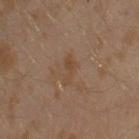notes: imaged on a skin check; not biopsied
body site: the arm
patient: male, roughly 30 years of age
imaging modality: total-body-photography crop, ~15 mm field of view